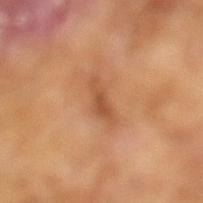This lesion was catalogued during total-body skin photography and was not selected for biopsy. Approximately 4 mm at its widest. The total-body-photography lesion software estimated an area of roughly 4.5 mm², a shape eccentricity near 0.95, and two-axis asymmetry of about 0.4. The software also gave roughly 9 lightness units darker than nearby skin. This image is a 15 mm lesion crop taken from a total-body photograph. A male patient about 65 years old.